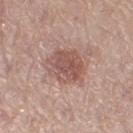The lesion was photographed on a routine skin check and not biopsied; there is no pathology result.
A 15 mm crop from a total-body photograph taken for skin-cancer surveillance.
A female patient approximately 65 years of age.
Captured under white-light illumination.
Located on the left thigh.